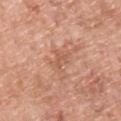Located on the back. Automated tile analysis of the lesion measured a lesion color around L≈58 a*≈23 b*≈33 in CIELAB, roughly 7 lightness units darker than nearby skin, and a normalized lesion–skin contrast near 5. The software also gave a classifier nevus-likeness of about 0/100 and a detector confidence of about 100 out of 100 that the crop contains a lesion. The patient is a male aged 53–57. A 15 mm close-up extracted from a 3D total-body photography capture.